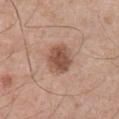The lesion was photographed on a routine skin check and not biopsied; there is no pathology result.
The tile uses white-light illumination.
Located on the abdomen.
A lesion tile, about 15 mm wide, cut from a 3D total-body photograph.
Automated image analysis of the tile measured an area of roughly 10 mm² and an eccentricity of roughly 0.6. The analysis additionally found an average lesion color of about L≈52 a*≈20 b*≈28 (CIELAB) and a lesion–skin lightness drop of about 12. The software also gave a within-lesion color-variation index near 3.5/10 and radial color variation of about 1.5. It also reported a detector confidence of about 100 out of 100 that the crop contains a lesion.
Measured at roughly 4 mm in maximum diameter.
A male patient, in their 70s.